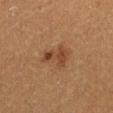follow-up: catalogued during a skin exam; not biopsied
body site: the right thigh
acquisition: 15 mm crop, total-body photography
image-analysis metrics: a lesion–skin lightness drop of about 8 and a lesion-to-skin contrast of about 7 (normalized; higher = more distinct); a within-lesion color-variation index near 2.5/10 and a peripheral color-asymmetry measure near 1; a classifier nevus-likeness of about 45/100 and a lesion-detection confidence of about 100/100
illumination: cross-polarized
patient: female, in their 40s
lesion diameter: about 4 mm